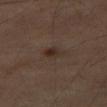No biopsy was performed on this lesion — it was imaged during a full skin examination and was not determined to be concerning. A male patient, aged approximately 65. From the left leg. Cropped from a whole-body photographic skin survey; the tile spans about 15 mm.